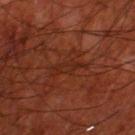notes = total-body-photography surveillance lesion; no biopsy | subject = male, about 70 years old | image source = ~15 mm crop, total-body skin-cancer survey | site = the left thigh.